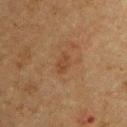Clinical impression:
The lesion was photographed on a routine skin check and not biopsied; there is no pathology result.
Context:
A lesion tile, about 15 mm wide, cut from a 3D total-body photograph. About 3 mm across. A male subject in their mid- to late 70s. Located on the front of the torso. Imaged with cross-polarized lighting.